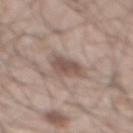Located on the back. Captured under white-light illumination. A 15 mm close-up extracted from a 3D total-body photography capture. The lesion-visualizer software estimated a footprint of about 7 mm², a shape eccentricity near 0.75, and a symmetry-axis asymmetry near 0.3. And it measured a normalized lesion–skin contrast near 7.5. It also reported a border-irregularity index near 3/10 and peripheral color asymmetry of about 1. And it measured a nevus-likeness score of about 50/100 and a lesion-detection confidence of about 95/100. A male patient, aged 48 to 52.